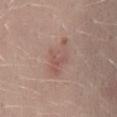Findings:
– biopsy status: total-body-photography surveillance lesion; no biopsy
– imaging modality: 15 mm crop, total-body photography
– patient: male, aged 28–32
– lesion size: ~5 mm (longest diameter)